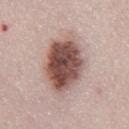automated metrics: a mean CIELAB color near L≈52 a*≈20 b*≈22, a lesion–skin lightness drop of about 19, and a normalized border contrast of about 12; a border-irregularity rating of about 2/10, a color-variation rating of about 8.5/10, and a peripheral color-asymmetry measure near 3 | patient: female, about 50 years old | body site: the chest | image: 15 mm crop, total-body photography | tile lighting: white-light | lesion diameter: ~8 mm (longest diameter).